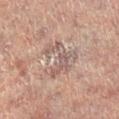{"image": {"source": "total-body photography crop", "field_of_view_mm": 15}, "lighting": "cross-polarized", "site": "left leg", "lesion_size": {"long_diameter_mm_approx": 5.0}, "patient": {"sex": "female", "age_approx": 80}}